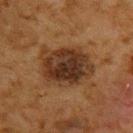Part of a total-body skin-imaging series; this lesion was reviewed on a skin check and was not flagged for biopsy.
Located on the back.
A lesion tile, about 15 mm wide, cut from a 3D total-body photograph.
A male patient, approximately 60 years of age.
Automated tile analysis of the lesion measured a mean CIELAB color near L≈27 a*≈16 b*≈26 and a normalized lesion–skin contrast near 11. The analysis additionally found a border-irregularity index near 2/10, a within-lesion color-variation index near 6/10, and a peripheral color-asymmetry measure near 2.
Measured at roughly 6 mm in maximum diameter.